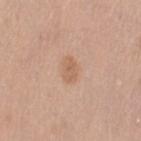Q: Was a biopsy performed?
A: imaged on a skin check; not biopsied
Q: What are the patient's age and sex?
A: female, aged 38–42
Q: Lesion location?
A: the left thigh
Q: What kind of image is this?
A: total-body-photography crop, ~15 mm field of view